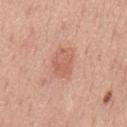Clinical impression: Captured during whole-body skin photography for melanoma surveillance; the lesion was not biopsied. Background: The recorded lesion diameter is about 4 mm. A 15 mm close-up extracted from a 3D total-body photography capture. A male subject, approximately 50 years of age. From the mid back.